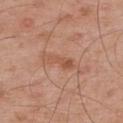Automated tile analysis of the lesion measured a mean CIELAB color near L≈54 a*≈23 b*≈32. The software also gave internal color variation of about 2 on a 0–10 scale and peripheral color asymmetry of about 0.5. The software also gave an automated nevus-likeness rating near 0 out of 100.
A 15 mm crop from a total-body photograph taken for skin-cancer surveillance.
On the back.
A male patient, in their mid- to late 50s.